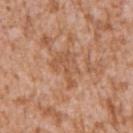The lesion was tiled from a total-body skin photograph and was not biopsied. The lesion is on the left upper arm. A male patient, approximately 45 years of age. Automated tile analysis of the lesion measured a lesion color around L≈55 a*≈22 b*≈34 in CIELAB and a normalized lesion–skin contrast near 5.5. And it measured a border-irregularity index near 7.5/10. Measured at roughly 4.5 mm in maximum diameter. This is a white-light tile. A region of skin cropped from a whole-body photographic capture, roughly 15 mm wide.